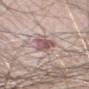biopsy status: total-body-photography surveillance lesion; no biopsy | TBP lesion metrics: an average lesion color of about L≈56 a*≈19 b*≈18 (CIELAB), roughly 12 lightness units darker than nearby skin, and a normalized lesion–skin contrast near 8; a nevus-likeness score of about 15/100 | site: the right thigh | patient: male, aged approximately 60 | acquisition: ~15 mm tile from a whole-body skin photo | lighting: white-light illumination | lesion size: ≈3.5 mm.